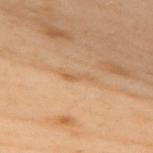Notes:
• patient · female, roughly 60 years of age
• anatomic site · the back
• TBP lesion metrics · an area of roughly 3 mm², a shape eccentricity near 0.95, and a shape-asymmetry score of about 0.4 (0 = symmetric); border irregularity of about 5 on a 0–10 scale, a within-lesion color-variation index near 0/10, and peripheral color asymmetry of about 0
• imaging modality · total-body-photography crop, ~15 mm field of view
• diameter · ≈3.5 mm
• tile lighting · cross-polarized illumination